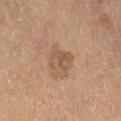follow-up — total-body-photography surveillance lesion; no biopsy
body site — the right thigh
subject — female, aged 78 to 82
diameter — ≈3 mm
image source — total-body-photography crop, ~15 mm field of view